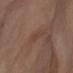Case summary:
* biopsy status · total-body-photography surveillance lesion; no biopsy
* body site · the abdomen
* lesion diameter · ≈3.5 mm
* automated metrics · a lesion color around L≈41 a*≈19 b*≈27 in CIELAB; a nevus-likeness score of about 0/100 and a detector confidence of about 50 out of 100 that the crop contains a lesion
* subject · female, roughly 55 years of age
* image source · 15 mm crop, total-body photography
* lighting · cross-polarized